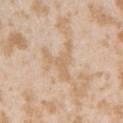The lesion was photographed on a routine skin check and not biopsied; there is no pathology result. The tile uses white-light illumination. The recorded lesion diameter is about 4.5 mm. A female patient, aged around 25. A lesion tile, about 15 mm wide, cut from a 3D total-body photograph. The lesion is located on the arm.